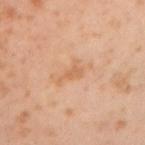Q: What kind of image is this?
A: ~15 mm crop, total-body skin-cancer survey
Q: Lesion location?
A: the right upper arm
Q: Patient demographics?
A: male, approximately 55 years of age